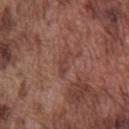imaging modality: total-body-photography crop, ~15 mm field of view
illumination: white-light
lesion diameter: about 3 mm
site: the chest
subject: male, in their mid-70s